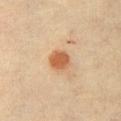Case summary:
– notes · imaged on a skin check; not biopsied
– diameter · ~3 mm (longest diameter)
– tile lighting · cross-polarized
– image · ~15 mm crop, total-body skin-cancer survey
– subject · male, roughly 40 years of age
– anatomic site · the right upper arm
– TBP lesion metrics · a lesion area of about 6 mm², a shape eccentricity near 0.45, and a shape-asymmetry score of about 0.2 (0 = symmetric); an average lesion color of about L≈53 a*≈21 b*≈34 (CIELAB), about 11 CIELAB-L* units darker than the surrounding skin, and a normalized border contrast of about 8.5; an automated nevus-likeness rating near 100 out of 100